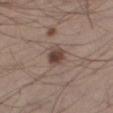Case summary:
• biopsy status — catalogued during a skin exam; not biopsied
• lesion size — about 3 mm
• image — ~15 mm tile from a whole-body skin photo
• illumination — white-light
• patient — male, in their mid- to late 30s
• automated lesion analysis — an outline eccentricity of about 0.55 (0 = round, 1 = elongated) and two-axis asymmetry of about 0.25; border irregularity of about 2.5 on a 0–10 scale, a color-variation rating of about 4.5/10, and peripheral color asymmetry of about 1.5
• site — the right thigh